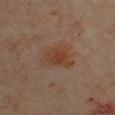Q: Was a biopsy performed?
A: imaged on a skin check; not biopsied
Q: How was this image acquired?
A: 15 mm crop, total-body photography
Q: Where on the body is the lesion?
A: the front of the torso
Q: Patient demographics?
A: female, aged 38 to 42